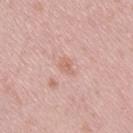Recorded during total-body skin imaging; not selected for excision or biopsy.
The lesion-visualizer software estimated border irregularity of about 3 on a 0–10 scale, internal color variation of about 1 on a 0–10 scale, and peripheral color asymmetry of about 0.5. And it measured a classifier nevus-likeness of about 0/100 and a lesion-detection confidence of about 100/100.
The subject is a female aged 28 to 32.
A lesion tile, about 15 mm wide, cut from a 3D total-body photograph.
On the right thigh.